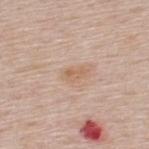Captured under white-light illumination. About 2.5 mm across. From the back. Cropped from a total-body skin-imaging series; the visible field is about 15 mm. A male subject, aged around 60.